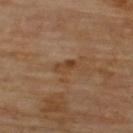Imaged during a routine full-body skin examination; the lesion was not biopsied and no histopathology is available. Imaged with cross-polarized lighting. Measured at roughly 3 mm in maximum diameter. A close-up tile cropped from a whole-body skin photograph, about 15 mm across. A male subject aged approximately 70. On the upper back.